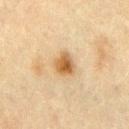<lesion>
<site>front of the torso</site>
<automated_metrics>
  <area_mm2_approx>6.0</area_mm2_approx>
  <eccentricity>0.45</eccentricity>
  <shape_asymmetry>0.25</shape_asymmetry>
  <border_irregularity_0_10>2.0</border_irregularity_0_10>
  <color_variation_0_10>5.0</color_variation_0_10>
  <peripheral_color_asymmetry>1.5</peripheral_color_asymmetry>
</automated_metrics>
<image>
  <source>total-body photography crop</source>
  <field_of_view_mm>15</field_of_view_mm>
</image>
<patient>
  <sex>male</sex>
  <age_approx>70</age_approx>
</patient>
<lighting>cross-polarized</lighting>
</lesion>